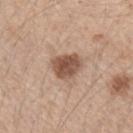The lesion was tiled from a total-body skin photograph and was not biopsied.
Captured under white-light illumination.
A close-up tile cropped from a whole-body skin photograph, about 15 mm across.
The lesion's longest dimension is about 3.5 mm.
The total-body-photography lesion software estimated a footprint of about 9 mm², an outline eccentricity of about 0.4 (0 = round, 1 = elongated), and two-axis asymmetry of about 0.2.
Located on the left forearm.
The patient is a female aged around 45.